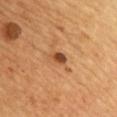Findings:
* notes: no biopsy performed (imaged during a skin exam)
* patient: male, about 45 years old
* automated metrics: a lesion area of about 2.5 mm², a shape eccentricity near 0.8, and two-axis asymmetry of about 0.2; a lesion color around L≈46 a*≈24 b*≈37 in CIELAB, about 15 CIELAB-L* units darker than the surrounding skin, and a normalized lesion–skin contrast near 10.5; a border-irregularity rating of about 1.5/10, internal color variation of about 4 on a 0–10 scale, and radial color variation of about 1.5
* imaging modality: 15 mm crop, total-body photography
* diameter: about 2 mm
* body site: the chest
* tile lighting: cross-polarized